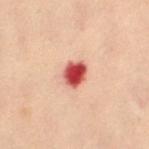Clinical impression: Recorded during total-body skin imaging; not selected for excision or biopsy. Background: Captured under cross-polarized illumination. A female patient, about 60 years old. The lesion-visualizer software estimated a footprint of about 6 mm² and a symmetry-axis asymmetry near 0.15. The analysis additionally found a mean CIELAB color near L≈47 a*≈34 b*≈27 and a normalized border contrast of about 13. And it measured a classifier nevus-likeness of about 0/100 and lesion-presence confidence of about 100/100. From the abdomen. The lesion's longest dimension is about 3 mm. Cropped from a total-body skin-imaging series; the visible field is about 15 mm.